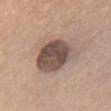The lesion was tiled from a total-body skin photograph and was not biopsied.